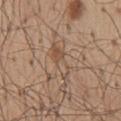Case summary:
* biopsy status — total-body-photography surveillance lesion; no biopsy
* body site — the mid back
* illumination — white-light illumination
* patient — male, in their mid- to late 50s
* lesion diameter — ≈5 mm
* TBP lesion metrics — a lesion color around L≈52 a*≈16 b*≈28 in CIELAB, about 7 CIELAB-L* units darker than the surrounding skin, and a normalized border contrast of about 5; a border-irregularity rating of about 4/10 and a peripheral color-asymmetry measure near 2.5; a nevus-likeness score of about 0/100
* acquisition — ~15 mm crop, total-body skin-cancer survey